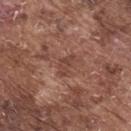Background:
Automated image analysis of the tile measured a lesion area of about 3.5 mm², an outline eccentricity of about 0.85 (0 = round, 1 = elongated), and a symmetry-axis asymmetry near 0.4. The software also gave a lesion color around L≈43 a*≈22 b*≈26 in CIELAB and a normalized border contrast of about 6. It also reported a border-irregularity rating of about 4/10, a within-lesion color-variation index near 2/10, and radial color variation of about 0.5. A male subject, about 75 years old. This is a white-light tile. A 15 mm close-up extracted from a 3D total-body photography capture. Approximately 3 mm at its widest. From the upper back.